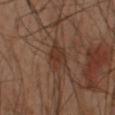Q: Is there a histopathology result?
A: imaged on a skin check; not biopsied
Q: What kind of image is this?
A: 15 mm crop, total-body photography
Q: How was the tile lit?
A: cross-polarized
Q: Who is the patient?
A: male, approximately 50 years of age
Q: Automated lesion metrics?
A: a lesion area of about 6.5 mm² and an eccentricity of roughly 0.25; a lesion–skin lightness drop of about 6 and a normalized lesion–skin contrast near 6.5; an automated nevus-likeness rating near 5 out of 100 and lesion-presence confidence of about 100/100
Q: What is the lesion's diameter?
A: ~3 mm (longest diameter)
Q: Where on the body is the lesion?
A: the left arm